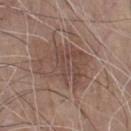No biopsy was performed on this lesion — it was imaged during a full skin examination and was not determined to be concerning.
Imaged with white-light lighting.
About 6 mm across.
The lesion-visualizer software estimated a lesion color around L≈47 a*≈17 b*≈23 in CIELAB, a lesion–skin lightness drop of about 8, and a normalized lesion–skin contrast near 6. It also reported a border-irregularity rating of about 5/10, a within-lesion color-variation index near 4.5/10, and radial color variation of about 1.5.
A male patient approximately 80 years of age.
A close-up tile cropped from a whole-body skin photograph, about 15 mm across.
The lesion is located on the chest.